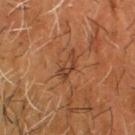Case summary:
– workup: catalogued during a skin exam; not biopsied
– lighting: cross-polarized
– acquisition: ~15 mm tile from a whole-body skin photo
– anatomic site: the head or neck
– lesion diameter: about 3.5 mm
– patient: male, aged around 65
– automated metrics: a mean CIELAB color near L≈39 a*≈22 b*≈32 and roughly 8 lightness units darker than nearby skin; an automated nevus-likeness rating near 0 out of 100